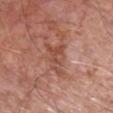Imaged during a routine full-body skin examination; the lesion was not biopsied and no histopathology is available. A male patient, approximately 65 years of age. The lesion is on the chest. A 15 mm crop from a total-body photograph taken for skin-cancer surveillance.